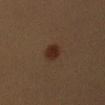Notes:
* follow-up: imaged on a skin check; not biopsied
* lighting: cross-polarized illumination
* subject: female, approximately 40 years of age
* acquisition: total-body-photography crop, ~15 mm field of view
* lesion diameter: about 3 mm
* site: the left upper arm